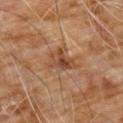Clinical impression:
Captured during whole-body skin photography for melanoma surveillance; the lesion was not biopsied.
Clinical summary:
About 3.5 mm across. On the chest. Imaged with cross-polarized lighting. This image is a 15 mm lesion crop taken from a total-body photograph. A male patient approximately 60 years of age. An algorithmic analysis of the crop reported a lesion color around L≈44 a*≈21 b*≈32 in CIELAB, about 10 CIELAB-L* units darker than the surrounding skin, and a normalized lesion–skin contrast near 7.5. The software also gave a classifier nevus-likeness of about 0/100 and lesion-presence confidence of about 100/100.